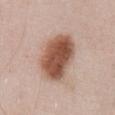The lesion was tiled from a total-body skin photograph and was not biopsied. From the abdomen. The tile uses white-light illumination. A male patient in their mid- to late 50s. About 6.5 mm across. This image is a 15 mm lesion crop taken from a total-body photograph.